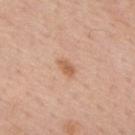Clinical impression:
No biopsy was performed on this lesion — it was imaged during a full skin examination and was not determined to be concerning.
Acquisition and patient details:
The lesion is located on the upper back. The total-body-photography lesion software estimated a lesion area of about 3 mm², an outline eccentricity of about 0.85 (0 = round, 1 = elongated), and a shape-asymmetry score of about 0.3 (0 = symmetric). And it measured a lesion color around L≈60 a*≈22 b*≈34 in CIELAB and a normalized border contrast of about 7. It also reported a classifier nevus-likeness of about 70/100 and a detector confidence of about 100 out of 100 that the crop contains a lesion. A 15 mm close-up extracted from a 3D total-body photography capture. The subject is a male approximately 60 years of age. The lesion's longest dimension is about 2.5 mm.